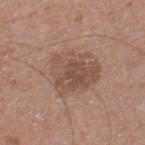A male subject, aged around 50.
The lesion's longest dimension is about 6.5 mm.
From the right lower leg.
Captured under white-light illumination.
A region of skin cropped from a whole-body photographic capture, roughly 15 mm wide.